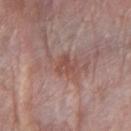Clinical impression:
Captured during whole-body skin photography for melanoma surveillance; the lesion was not biopsied.
Image and clinical context:
Longest diameter approximately 2.5 mm. Imaged with white-light lighting. Automated tile analysis of the lesion measured an automated nevus-likeness rating near 0 out of 100 and a lesion-detection confidence of about 100/100. The lesion is located on the right forearm. This image is a 15 mm lesion crop taken from a total-body photograph. The patient is a female aged 63 to 67.